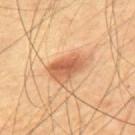This lesion was catalogued during total-body skin photography and was not selected for biopsy.
Longest diameter approximately 5 mm.
This is a cross-polarized tile.
A male patient approximately 65 years of age.
A 15 mm close-up tile from a total-body photography series done for melanoma screening.
From the mid back.